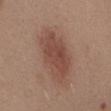Assessment:
Imaged during a routine full-body skin examination; the lesion was not biopsied and no histopathology is available.
Clinical summary:
From the abdomen. A female subject aged approximately 40. This image is a 15 mm lesion crop taken from a total-body photograph.